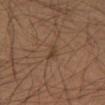The lesion is located on the leg. The patient is a male roughly 40 years of age. A 15 mm crop from a total-body photograph taken for skin-cancer surveillance.